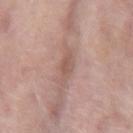The lesion was tiled from a total-body skin photograph and was not biopsied. Imaged with white-light lighting. The lesion is located on the right forearm. Cropped from a whole-body photographic skin survey; the tile spans about 15 mm. The patient is a female aged 68–72. An algorithmic analysis of the crop reported a lesion color around L≈56 a*≈19 b*≈25 in CIELAB and a normalized lesion–skin contrast near 5.5. And it measured a border-irregularity index near 3/10, a color-variation rating of about 2/10, and a peripheral color-asymmetry measure near 0.5. The software also gave a nevus-likeness score of about 0/100 and a detector confidence of about 100 out of 100 that the crop contains a lesion.